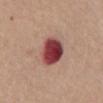<lesion>
<biopsy_status>not biopsied; imaged during a skin examination</biopsy_status>
<image>
  <source>total-body photography crop</source>
  <field_of_view_mm>15</field_of_view_mm>
</image>
<site>abdomen</site>
<patient>
  <sex>male</sex>
  <age_approx>65</age_approx>
</patient>
</lesion>